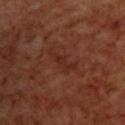follow-up: no biopsy performed (imaged during a skin exam) | lighting: cross-polarized illumination | anatomic site: the upper back | diameter: ≈4 mm | image: ~15 mm crop, total-body skin-cancer survey | patient: male, about 70 years old.